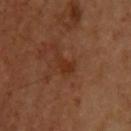<tbp_lesion>
<lighting>cross-polarized</lighting>
<image>
  <source>total-body photography crop</source>
  <field_of_view_mm>15</field_of_view_mm>
</image>
<lesion_size>
  <long_diameter_mm_approx>2.5</long_diameter_mm_approx>
</lesion_size>
<automated_metrics>
  <area_mm2_approx>3.0</area_mm2_approx>
  <shape_asymmetry>0.35</shape_asymmetry>
  <cielab_L>32</cielab_L>
  <cielab_a>24</cielab_a>
  <cielab_b>32</cielab_b>
  <border_irregularity_0_10>4.0</border_irregularity_0_10>
  <color_variation_0_10>1.0</color_variation_0_10>
  <peripheral_color_asymmetry>0.5</peripheral_color_asymmetry>
</automated_metrics>
<site>upper back</site>
</tbp_lesion>